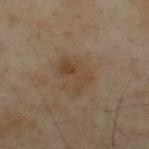Acquisition and patient details: On the upper back. A male patient aged 53–57. Cropped from a whole-body photographic skin survey; the tile spans about 15 mm.